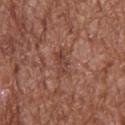From the back. About 4 mm across. A male patient approximately 75 years of age. The tile uses white-light illumination. A close-up tile cropped from a whole-body skin photograph, about 15 mm across.